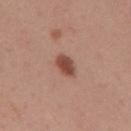Q: Is there a histopathology result?
A: imaged on a skin check; not biopsied
Q: How was this image acquired?
A: ~15 mm tile from a whole-body skin photo
Q: How large is the lesion?
A: ~3 mm (longest diameter)
Q: What are the patient's age and sex?
A: female, roughly 50 years of age
Q: Illumination type?
A: white-light illumination
Q: Automated lesion metrics?
A: an average lesion color of about L≈47 a*≈23 b*≈28 (CIELAB), roughly 13 lightness units darker than nearby skin, and a lesion-to-skin contrast of about 9.5 (normalized; higher = more distinct)
Q: What is the anatomic site?
A: the back